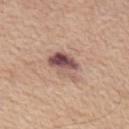No biopsy was performed on this lesion — it was imaged during a full skin examination and was not determined to be concerning. A region of skin cropped from a whole-body photographic capture, roughly 15 mm wide. The subject is a male aged around 55. Longest diameter approximately 4.5 mm. The lesion-visualizer software estimated a lesion area of about 9 mm², an eccentricity of roughly 0.8, and a shape-asymmetry score of about 0.25 (0 = symmetric). The analysis additionally found a within-lesion color-variation index near 10/10 and radial color variation of about 6. From the chest.